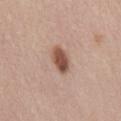Assessment: No biopsy was performed on this lesion — it was imaged during a full skin examination and was not determined to be concerning. Context: A 15 mm crop from a total-body photograph taken for skin-cancer surveillance. Approximately 4 mm at its widest. Captured under white-light illumination. On the mid back. The patient is a female in their 50s.